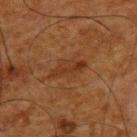Case summary:
• follow-up — no biopsy performed (imaged during a skin exam)
• acquisition — ~15 mm crop, total-body skin-cancer survey
• subject — male, in their mid- to late 60s
• body site — the upper back
• lighting — cross-polarized
• diameter — ≈5 mm